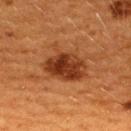Clinical impression: Recorded during total-body skin imaging; not selected for excision or biopsy. Context: Imaged with cross-polarized lighting. A female patient aged approximately 40. The recorded lesion diameter is about 5.5 mm. From the upper back. A lesion tile, about 15 mm wide, cut from a 3D total-body photograph.